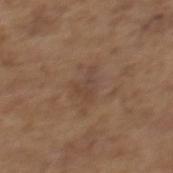Part of a total-body skin-imaging series; this lesion was reviewed on a skin check and was not flagged for biopsy.
Automated image analysis of the tile measured a lesion color around L≈43 a*≈17 b*≈26 in CIELAB, about 6 CIELAB-L* units darker than the surrounding skin, and a lesion-to-skin contrast of about 5 (normalized; higher = more distinct). The software also gave a border-irregularity index near 5/10, a within-lesion color-variation index near 2/10, and radial color variation of about 0.5.
This is a white-light tile.
A region of skin cropped from a whole-body photographic capture, roughly 15 mm wide.
A female patient, approximately 65 years of age.
The lesion's longest dimension is about 3.5 mm.
The lesion is on the mid back.